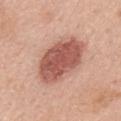{
  "image": {
    "source": "total-body photography crop",
    "field_of_view_mm": 15
  },
  "site": "mid back",
  "patient": {
    "sex": "female",
    "age_approx": 60
  }
}